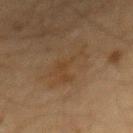Impression:
Imaged during a routine full-body skin examination; the lesion was not biopsied and no histopathology is available.
Context:
A male patient approximately 60 years of age. A region of skin cropped from a whole-body photographic capture, roughly 15 mm wide. On the back. Captured under cross-polarized illumination. An algorithmic analysis of the crop reported a footprint of about 6.5 mm², a shape eccentricity near 0.9, and a symmetry-axis asymmetry near 0.75. It also reported a border-irregularity index near 10/10 and peripheral color asymmetry of about 0. The software also gave an automated nevus-likeness rating near 0 out of 100 and lesion-presence confidence of about 100/100.